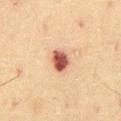Notes:
- biopsy status: total-body-photography surveillance lesion; no biopsy
- imaging modality: ~15 mm crop, total-body skin-cancer survey
- lesion diameter: ~3 mm (longest diameter)
- illumination: cross-polarized
- subject: male, about 60 years old
- location: the abdomen